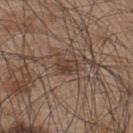Q: Was a biopsy performed?
A: total-body-photography surveillance lesion; no biopsy
Q: Lesion size?
A: about 3.5 mm
Q: What kind of image is this?
A: ~15 mm crop, total-body skin-cancer survey
Q: Who is the patient?
A: male, aged approximately 45
Q: What did automated image analysis measure?
A: an area of roughly 5.5 mm² and an eccentricity of roughly 0.75; a border-irregularity index near 4.5/10 and radial color variation of about 1.5; a lesion-detection confidence of about 95/100
Q: Lesion location?
A: the back